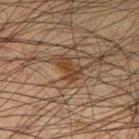Assessment: The lesion was tiled from a total-body skin photograph and was not biopsied. Image and clinical context: Imaged with cross-polarized lighting. Automated image analysis of the tile measured a nevus-likeness score of about 95/100 and lesion-presence confidence of about 100/100. A region of skin cropped from a whole-body photographic capture, roughly 15 mm wide. Located on the leg. A male subject roughly 45 years of age.